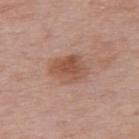| feature | finding |
|---|---|
| workup | imaged on a skin check; not biopsied |
| automated metrics | a lesion area of about 10 mm², a shape eccentricity near 0.75, and a symmetry-axis asymmetry near 0.2; a color-variation rating of about 4/10 and radial color variation of about 1; an automated nevus-likeness rating near 55 out of 100 and lesion-presence confidence of about 100/100 |
| acquisition | ~15 mm tile from a whole-body skin photo |
| patient | female, about 50 years old |
| size | ~4.5 mm (longest diameter) |
| site | the back |
| lighting | white-light |